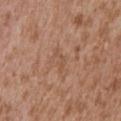Captured during whole-body skin photography for melanoma surveillance; the lesion was not biopsied.
A lesion tile, about 15 mm wide, cut from a 3D total-body photograph.
Located on the abdomen.
A male subject aged 43 to 47.